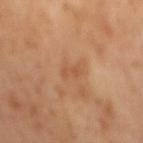illumination = cross-polarized illumination | automated metrics = a border-irregularity index near 4.5/10, a color-variation rating of about 0/10, and radial color variation of about 0 | diameter = about 2.5 mm | imaging modality = ~15 mm tile from a whole-body skin photo | subject = male, in their mid-60s.